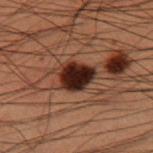No biopsy was performed on this lesion — it was imaged during a full skin examination and was not determined to be concerning. The recorded lesion diameter is about 3.5 mm. A 15 mm crop from a total-body photograph taken for skin-cancer surveillance. A male subject, aged 48 to 52. Located on the leg. Captured under cross-polarized illumination. Automated tile analysis of the lesion measured a lesion color around L≈18 a*≈17 b*≈19 in CIELAB and a lesion-to-skin contrast of about 18.5 (normalized; higher = more distinct). It also reported a border-irregularity rating of about 2/10, internal color variation of about 5 on a 0–10 scale, and radial color variation of about 1. And it measured a nevus-likeness score of about 65/100 and a detector confidence of about 100 out of 100 that the crop contains a lesion.